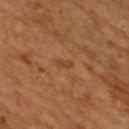{
  "biopsy_status": "not biopsied; imaged during a skin examination"
}